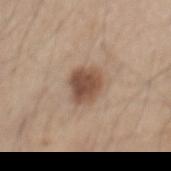Case summary:
* biopsy status — no biopsy performed (imaged during a skin exam)
* imaging modality — 15 mm crop, total-body photography
* automated metrics — a lesion area of about 9 mm², a shape eccentricity near 0.45, and two-axis asymmetry of about 0.15; a classifier nevus-likeness of about 95/100 and a detector confidence of about 100 out of 100 that the crop contains a lesion
* tile lighting — white-light
* location — the mid back
* patient — male, in their mid-40s